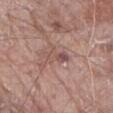Imaged during a routine full-body skin examination; the lesion was not biopsied and no histopathology is available.
A lesion tile, about 15 mm wide, cut from a 3D total-body photograph.
A male subject aged around 80.
The total-body-photography lesion software estimated an area of roughly 4.5 mm², an outline eccentricity of about 0.9 (0 = round, 1 = elongated), and two-axis asymmetry of about 0.3.
The tile uses white-light illumination.
Approximately 3.5 mm at its widest.
The lesion is on the front of the torso.